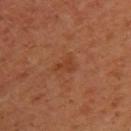No biopsy was performed on this lesion — it was imaged during a full skin examination and was not determined to be concerning.
Automated image analysis of the tile measured a border-irregularity rating of about 5/10, a within-lesion color-variation index near 0.5/10, and a peripheral color-asymmetry measure near 0. And it measured a lesion-detection confidence of about 100/100.
The lesion is on the upper back.
Captured under cross-polarized illumination.
A 15 mm close-up tile from a total-body photography series done for melanoma screening.
Measured at roughly 2.5 mm in maximum diameter.
The subject is a female aged approximately 40.